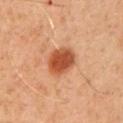follow-up: total-body-photography surveillance lesion; no biopsy | body site: the chest | lesion size: about 4 mm | subject: male, roughly 50 years of age | tile lighting: cross-polarized illumination | image source: ~15 mm tile from a whole-body skin photo.